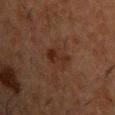Q: Was a biopsy performed?
A: imaged on a skin check; not biopsied
Q: Lesion size?
A: about 3.5 mm
Q: What did automated image analysis measure?
A: an area of roughly 5.5 mm² and a shape-asymmetry score of about 0.4 (0 = symmetric); a nevus-likeness score of about 25/100 and a detector confidence of about 100 out of 100 that the crop contains a lesion
Q: Illumination type?
A: cross-polarized illumination
Q: What are the patient's age and sex?
A: male, aged 48–52
Q: What kind of image is this?
A: total-body-photography crop, ~15 mm field of view
Q: What is the anatomic site?
A: the chest